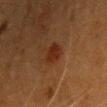| key | value |
|---|---|
| notes | no biopsy performed (imaged during a skin exam) |
| image source | total-body-photography crop, ~15 mm field of view |
| illumination | cross-polarized illumination |
| diameter | ~2.5 mm (longest diameter) |
| location | the front of the torso |
| automated metrics | a footprint of about 4.5 mm² and two-axis asymmetry of about 0.25; a mean CIELAB color near L≈24 a*≈22 b*≈27 and a normalized lesion–skin contrast near 8; a border-irregularity index near 2/10 and internal color variation of about 2 on a 0–10 scale; a classifier nevus-likeness of about 95/100 and lesion-presence confidence of about 100/100 |
| subject | female, roughly 40 years of age |